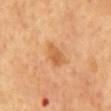Captured during whole-body skin photography for melanoma surveillance; the lesion was not biopsied.
Measured at roughly 3.5 mm in maximum diameter.
The subject is a male aged approximately 65.
Automated tile analysis of the lesion measured a footprint of about 5.5 mm², an outline eccentricity of about 0.85 (0 = round, 1 = elongated), and a shape-asymmetry score of about 0.3 (0 = symmetric). The analysis additionally found a mean CIELAB color near L≈61 a*≈25 b*≈43, a lesion–skin lightness drop of about 9, and a normalized lesion–skin contrast near 6.5. It also reported internal color variation of about 3 on a 0–10 scale and a peripheral color-asymmetry measure near 1. The software also gave a classifier nevus-likeness of about 25/100 and a detector confidence of about 100 out of 100 that the crop contains a lesion.
The tile uses cross-polarized illumination.
The lesion is located on the mid back.
This image is a 15 mm lesion crop taken from a total-body photograph.